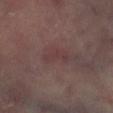| feature | finding |
|---|---|
| biopsy status | imaged on a skin check; not biopsied |